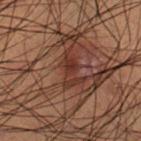{
  "biopsy_status": "not biopsied; imaged during a skin examination",
  "patient": {
    "sex": "male",
    "age_approx": 50
  },
  "lighting": "cross-polarized",
  "site": "right lower leg",
  "image": {
    "source": "total-body photography crop",
    "field_of_view_mm": 15
  },
  "automated_metrics": {
    "area_mm2_approx": 6.0,
    "shape_asymmetry": 0.35,
    "cielab_L": 26,
    "cielab_a": 18,
    "cielab_b": 21,
    "vs_skin_darker_L": 6.0,
    "vs_skin_contrast_norm": 7.0,
    "border_irregularity_0_10": 4.0,
    "color_variation_0_10": 3.5,
    "peripheral_color_asymmetry": 1.5
  },
  "lesion_size": {
    "long_diameter_mm_approx": 4.0
  }
}